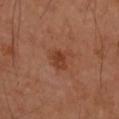Recorded during total-body skin imaging; not selected for excision or biopsy. The tile uses cross-polarized illumination. Automated tile analysis of the lesion measured a lesion area of about 4.5 mm², a shape eccentricity near 0.6, and two-axis asymmetry of about 0.25. And it measured lesion-presence confidence of about 100/100. A region of skin cropped from a whole-body photographic capture, roughly 15 mm wide. Located on the right upper arm. Longest diameter approximately 2.5 mm. A female patient aged approximately 55.